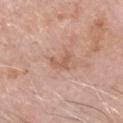Assessment: This lesion was catalogued during total-body skin photography and was not selected for biopsy. Clinical summary: Captured under white-light illumination. Approximately 2.5 mm at its widest. A male subject in their mid- to late 70s. A region of skin cropped from a whole-body photographic capture, roughly 15 mm wide. From the head or neck.